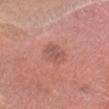This image is a 15 mm lesion crop taken from a total-body photograph.
This is a white-light tile.
An algorithmic analysis of the crop reported an area of roughly 7 mm², an outline eccentricity of about 0.65 (0 = round, 1 = elongated), and a shape-asymmetry score of about 0.3 (0 = symmetric). It also reported roughly 8 lightness units darker than nearby skin and a normalized border contrast of about 5.
The lesion is on the head or neck.
The patient is a male aged 28–32.
Measured at roughly 3.5 mm in maximum diameter.